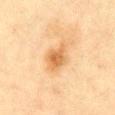Case summary:
• follow-up — catalogued during a skin exam; not biopsied
• location — the front of the torso
• lesion size — about 4.5 mm
• automated metrics — a footprint of about 8.5 mm², an eccentricity of roughly 0.75, and two-axis asymmetry of about 0.2; a mean CIELAB color near L≈57 a*≈19 b*≈38, about 10 CIELAB-L* units darker than the surrounding skin, and a normalized border contrast of about 7.5; a nevus-likeness score of about 65/100 and a detector confidence of about 100 out of 100 that the crop contains a lesion
• acquisition — ~15 mm crop, total-body skin-cancer survey
• subject — female, about 55 years old